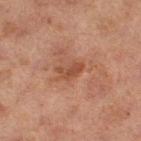Clinical impression:
Part of a total-body skin-imaging series; this lesion was reviewed on a skin check and was not flagged for biopsy.
Background:
A region of skin cropped from a whole-body photographic capture, roughly 15 mm wide. This is a cross-polarized tile. Located on the leg. The subject is a female approximately 60 years of age.